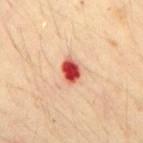biopsy status — no biopsy performed (imaged during a skin exam) | image-analysis metrics — a lesion area of about 5 mm², an eccentricity of roughly 0.7, and a symmetry-axis asymmetry near 0.25; an average lesion color of about L≈52 a*≈40 b*≈34 (CIELAB) and a normalized lesion–skin contrast near 14; a nevus-likeness score of about 0/100 and a lesion-detection confidence of about 100/100 | lesion diameter — about 3 mm | anatomic site — the mid back | acquisition — ~15 mm crop, total-body skin-cancer survey | subject — male, roughly 30 years of age.